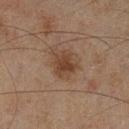Cropped from a whole-body photographic skin survey; the tile spans about 15 mm. Captured under cross-polarized illumination. Approximately 3 mm at its widest. The lesion is located on the left lower leg. A male subject, roughly 45 years of age.